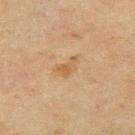An algorithmic analysis of the crop reported an area of roughly 3.5 mm², an outline eccentricity of about 0.85 (0 = round, 1 = elongated), and two-axis asymmetry of about 0.45. The software also gave a mean CIELAB color near L≈46 a*≈16 b*≈32 and a normalized border contrast of about 6.
A male subject aged 63 to 67.
Longest diameter approximately 3 mm.
Located on the arm.
A 15 mm crop from a total-body photograph taken for skin-cancer surveillance.
The tile uses cross-polarized illumination.